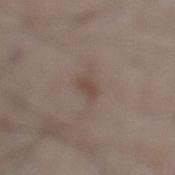| key | value |
|---|---|
| follow-up | no biopsy performed (imaged during a skin exam) |
| site | the left lower leg |
| imaging modality | ~15 mm tile from a whole-body skin photo |
| patient | male, aged around 50 |
| automated metrics | a footprint of about 2.5 mm², a shape eccentricity near 0.9, and a shape-asymmetry score of about 0.3 (0 = symmetric); a mean CIELAB color near L≈45 a*≈15 b*≈23, roughly 7 lightness units darker than nearby skin, and a normalized lesion–skin contrast near 6.5 |
| lighting | white-light illumination |